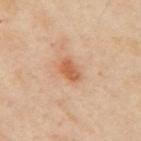Impression: Imaged during a routine full-body skin examination; the lesion was not biopsied and no histopathology is available. Background: The lesion is located on the left arm. A 15 mm close-up extracted from a 3D total-body photography capture. Captured under cross-polarized illumination. An algorithmic analysis of the crop reported an area of roughly 4 mm², a shape eccentricity near 0.8, and a shape-asymmetry score of about 0.25 (0 = symmetric). The software also gave a mean CIELAB color near L≈60 a*≈26 b*≈37, roughly 11 lightness units darker than nearby skin, and a normalized border contrast of about 7.5. The software also gave a color-variation rating of about 2.5/10 and peripheral color asymmetry of about 1. A female patient in their 40s.